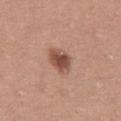Part of a total-body skin-imaging series; this lesion was reviewed on a skin check and was not flagged for biopsy.
About 3.5 mm across.
This is a white-light tile.
From the abdomen.
The subject is a female aged 48–52.
A lesion tile, about 15 mm wide, cut from a 3D total-body photograph.